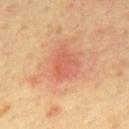The lesion was photographed on a routine skin check and not biopsied; there is no pathology result.
A male subject, aged around 65.
From the mid back.
A lesion tile, about 15 mm wide, cut from a 3D total-body photograph.